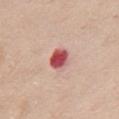{
  "site": "chest",
  "patient": {
    "sex": "female",
    "age_approx": 70
  },
  "image": {
    "source": "total-body photography crop",
    "field_of_view_mm": 15
  },
  "lighting": "white-light"
}